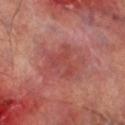Q: Where on the body is the lesion?
A: the leg
Q: What is the imaging modality?
A: total-body-photography crop, ~15 mm field of view
Q: What are the patient's age and sex?
A: male, approximately 70 years of age